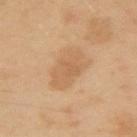Captured during whole-body skin photography for melanoma surveillance; the lesion was not biopsied. The tile uses cross-polarized illumination. A region of skin cropped from a whole-body photographic capture, roughly 15 mm wide. A male patient in their mid- to late 40s. The recorded lesion diameter is about 6 mm. An algorithmic analysis of the crop reported a lesion–skin lightness drop of about 7. On the arm.